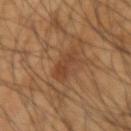lighting: cross-polarized
patient:
  sex: male
  age_approx: 65
site: arm
image:
  source: total-body photography crop
  field_of_view_mm: 15